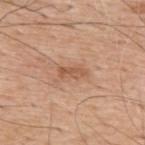{
  "biopsy_status": "not biopsied; imaged during a skin examination",
  "lighting": "white-light",
  "image": {
    "source": "total-body photography crop",
    "field_of_view_mm": 15
  },
  "lesion_size": {
    "long_diameter_mm_approx": 3.0
  },
  "patient": {
    "sex": "male",
    "age_approx": 60
  },
  "site": "upper back"
}